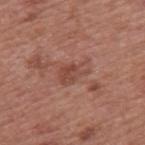Q: Is there a histopathology result?
A: no biopsy performed (imaged during a skin exam)